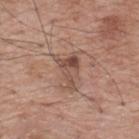| field | value |
|---|---|
| biopsy status | total-body-photography surveillance lesion; no biopsy |
| lesion size | ~4 mm (longest diameter) |
| acquisition | ~15 mm tile from a whole-body skin photo |
| site | the upper back |
| patient | male, about 70 years old |
| automated lesion analysis | an automated nevus-likeness rating near 5 out of 100 |